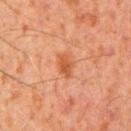Assessment:
Captured during whole-body skin photography for melanoma surveillance; the lesion was not biopsied.
Image and clinical context:
From the right upper arm. A male subject, roughly 35 years of age. A 15 mm crop from a total-body photograph taken for skin-cancer surveillance.